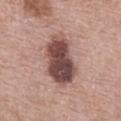Assessment: Recorded during total-body skin imaging; not selected for excision or biopsy. Clinical summary: From the chest. A male patient, in their 70s. A 15 mm crop from a total-body photograph taken for skin-cancer surveillance. Longest diameter approximately 7 mm.